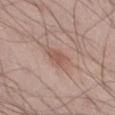patient: male, aged approximately 60 | illumination: white-light | image: 15 mm crop, total-body photography | anatomic site: the left thigh | lesion size: about 2.5 mm.